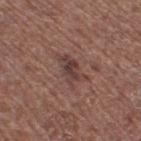<case>
  <biopsy_status>not biopsied; imaged during a skin examination</biopsy_status>
  <patient>
    <sex>female</sex>
    <age_approx>50</age_approx>
  </patient>
  <site>leg</site>
  <image>
    <source>total-body photography crop</source>
    <field_of_view_mm>15</field_of_view_mm>
  </image>
  <automated_metrics>
    <area_mm2_approx>7.5</area_mm2_approx>
    <eccentricity>0.85</eccentricity>
    <shape_asymmetry>0.4</shape_asymmetry>
    <cielab_L>40</cielab_L>
    <cielab_a>19</cielab_a>
    <cielab_b>21</cielab_b>
    <vs_skin_darker_L>8.0</vs_skin_darker_L>
    <vs_skin_contrast_norm>7.0</vs_skin_contrast_norm>
    <border_irregularity_0_10>5.5</border_irregularity_0_10>
    <color_variation_0_10>3.5</color_variation_0_10>
    <peripheral_color_asymmetry>1.0</peripheral_color_asymmetry>
  </automated_metrics>
  <lighting>white-light</lighting>
</case>